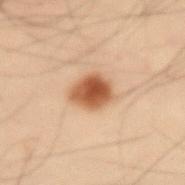  biopsy_status: not biopsied; imaged during a skin examination
  site: arm
  lighting: cross-polarized
  automated_metrics:
    area_mm2_approx: 9.0
    shape_asymmetry: 0.15
    color_variation_0_10: 4.5
    peripheral_color_asymmetry: 1.5
    nevus_likeness_0_100: 100
    lesion_detection_confidence_0_100: 100
  image:
    source: total-body photography crop
    field_of_view_mm: 15
  patient:
    sex: male
    age_approx: 55
  lesion_size:
    long_diameter_mm_approx: 3.5